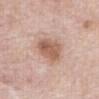| feature | finding |
|---|---|
| follow-up | total-body-photography surveillance lesion; no biopsy |
| automated lesion analysis | an area of roughly 10 mm², an eccentricity of roughly 0.65, and a shape-asymmetry score of about 0.25 (0 = symmetric); a classifier nevus-likeness of about 55/100 and a detector confidence of about 100 out of 100 that the crop contains a lesion |
| subject | female, approximately 65 years of age |
| illumination | white-light |
| lesion size | about 4 mm |
| site | the abdomen |
| imaging modality | ~15 mm tile from a whole-body skin photo |